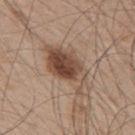The lesion was photographed on a routine skin check and not biopsied; there is no pathology result.
The lesion is located on the back.
Imaged with white-light lighting.
A male patient aged 48 to 52.
A roughly 15 mm field-of-view crop from a total-body skin photograph.
Automated tile analysis of the lesion measured a lesion area of about 17 mm², a shape eccentricity near 0.9, and a shape-asymmetry score of about 0.3 (0 = symmetric). It also reported an average lesion color of about L≈48 a*≈17 b*≈27 (CIELAB), roughly 13 lightness units darker than nearby skin, and a normalized lesion–skin contrast near 9.5. It also reported a border-irregularity rating of about 4.5/10, a within-lesion color-variation index near 8/10, and a peripheral color-asymmetry measure near 3.